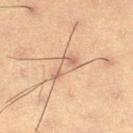Part of a total-body skin-imaging series; this lesion was reviewed on a skin check and was not flagged for biopsy.
About 3.5 mm across.
A male subject, in their mid-50s.
A 15 mm close-up extracted from a 3D total-body photography capture.
From the left thigh.